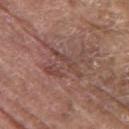Recorded during total-body skin imaging; not selected for excision or biopsy.
A 15 mm close-up extracted from a 3D total-body photography capture.
On the right upper arm.
The lesion's longest dimension is about 5 mm.
A male subject, aged around 80.
An algorithmic analysis of the crop reported a shape eccentricity near 0.75 and a symmetry-axis asymmetry near 0.45. And it measured an average lesion color of about L≈45 a*≈20 b*≈23 (CIELAB) and roughly 6 lightness units darker than nearby skin. The analysis additionally found a border-irregularity rating of about 8/10, internal color variation of about 4 on a 0–10 scale, and radial color variation of about 1.
Captured under white-light illumination.